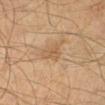Assessment:
This lesion was catalogued during total-body skin photography and was not selected for biopsy.
Acquisition and patient details:
From the right lower leg. This image is a 15 mm lesion crop taken from a total-body photograph. The subject is a male aged around 40.